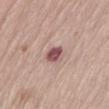Q: Is there a histopathology result?
A: no biopsy performed (imaged during a skin exam)
Q: What is the imaging modality?
A: ~15 mm crop, total-body skin-cancer survey
Q: How was the tile lit?
A: white-light illumination
Q: Patient demographics?
A: male, roughly 65 years of age
Q: What is the anatomic site?
A: the left thigh
Q: Lesion size?
A: ~2.5 mm (longest diameter)